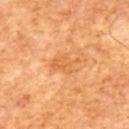Impression: Captured during whole-body skin photography for melanoma surveillance; the lesion was not biopsied. Acquisition and patient details: A lesion tile, about 15 mm wide, cut from a 3D total-body photograph. Captured under cross-polarized illumination. A male subject, roughly 65 years of age. Longest diameter approximately 3.5 mm.